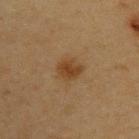Captured during whole-body skin photography for melanoma surveillance; the lesion was not biopsied. A region of skin cropped from a whole-body photographic capture, roughly 15 mm wide. Automated image analysis of the tile measured a footprint of about 6 mm², a shape eccentricity near 0.6, and a symmetry-axis asymmetry near 0.2. Imaged with cross-polarized lighting. The patient is a female aged 38 to 42. On the upper back. The lesion's longest dimension is about 3 mm.